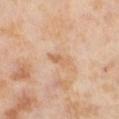Case summary:
• notes: total-body-photography surveillance lesion; no biopsy
• patient: female, in their mid-50s
• automated lesion analysis: a border-irregularity rating of about 3.5/10, a color-variation rating of about 0/10, and a peripheral color-asymmetry measure near 0; a nevus-likeness score of about 0/100 and a detector confidence of about 100 out of 100 that the crop contains a lesion
• size: about 3 mm
• site: the leg
• image source: 15 mm crop, total-body photography